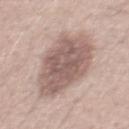Findings:
• tile lighting: white-light illumination
• size: ~8.5 mm (longest diameter)
• acquisition: ~15 mm crop, total-body skin-cancer survey
• location: the abdomen
• patient: male, in their 60s